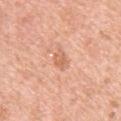{"biopsy_status": "not biopsied; imaged during a skin examination", "automated_metrics": {"cielab_L": 65, "cielab_a": 25, "cielab_b": 35, "vs_skin_contrast_norm": 6.0, "lesion_detection_confidence_0_100": 100}, "site": "left upper arm", "lesion_size": {"long_diameter_mm_approx": 2.5}, "lighting": "white-light", "image": {"source": "total-body photography crop", "field_of_view_mm": 15}, "patient": {"sex": "female", "age_approx": 40}}